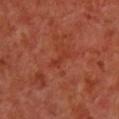Imaged during a routine full-body skin examination; the lesion was not biopsied and no histopathology is available.